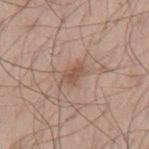notes = no biopsy performed (imaged during a skin exam) | imaging modality = ~15 mm crop, total-body skin-cancer survey | location = the mid back | subject = male, roughly 55 years of age.